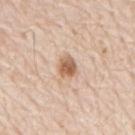From the mid back.
The recorded lesion diameter is about 3 mm.
The patient is a male roughly 80 years of age.
Cropped from a whole-body photographic skin survey; the tile spans about 15 mm.
Imaged with white-light lighting.